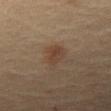Clinical impression: Captured during whole-body skin photography for melanoma surveillance; the lesion was not biopsied. Image and clinical context: This is a cross-polarized tile. A male patient aged approximately 65. The lesion's longest dimension is about 3 mm. The lesion is on the back. A close-up tile cropped from a whole-body skin photograph, about 15 mm across.